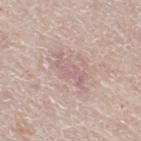The lesion's longest dimension is about 4.5 mm.
A 15 mm close-up extracted from a 3D total-body photography capture.
The lesion is on the right thigh.
The patient is a female aged 43–47.
Imaged with white-light lighting.